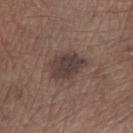notes — catalogued during a skin exam; not biopsied | location — the right forearm | patient — male, roughly 65 years of age | acquisition — ~15 mm crop, total-body skin-cancer survey | TBP lesion metrics — about 10 CIELAB-L* units darker than the surrounding skin and a normalized lesion–skin contrast near 9; a classifier nevus-likeness of about 10/100 and a detector confidence of about 100 out of 100 that the crop contains a lesion | lesion diameter — ~4.5 mm (longest diameter) | tile lighting — white-light illumination.